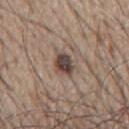<case>
  <site>mid back</site>
  <automated_metrics>
    <area_mm2_approx>5.5</area_mm2_approx>
    <eccentricity>0.75</eccentricity>
    <cielab_L>43</cielab_L>
    <cielab_a>14</cielab_a>
    <cielab_b>21</cielab_b>
    <vs_skin_darker_L>15.0</vs_skin_darker_L>
    <vs_skin_contrast_norm>11.5</vs_skin_contrast_norm>
    <border_irregularity_0_10>2.5</border_irregularity_0_10>
    <color_variation_0_10>6.0</color_variation_0_10>
  </automated_metrics>
  <image>
    <source>total-body photography crop</source>
    <field_of_view_mm>15</field_of_view_mm>
  </image>
  <lesion_size>
    <long_diameter_mm_approx>3.5</long_diameter_mm_approx>
  </lesion_size>
  <patient>
    <sex>male</sex>
    <age_approx>65</age_approx>
  </patient>
  <lighting>white-light</lighting>
</case>